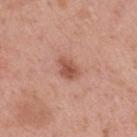Impression: Imaged during a routine full-body skin examination; the lesion was not biopsied and no histopathology is available. Clinical summary: The lesion is on the mid back. A region of skin cropped from a whole-body photographic capture, roughly 15 mm wide. The subject is a male aged approximately 40. Imaged with white-light lighting.